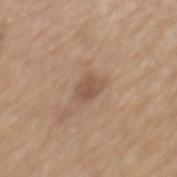From the mid back. A male patient, approximately 70 years of age. This is a white-light tile. A close-up tile cropped from a whole-body skin photograph, about 15 mm across. The recorded lesion diameter is about 2.5 mm.